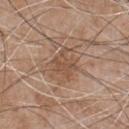The lesion was photographed on a routine skin check and not biopsied; there is no pathology result. A lesion tile, about 15 mm wide, cut from a 3D total-body photograph. The tile uses white-light illumination. On the chest. About 3.5 mm across. A male patient roughly 65 years of age.